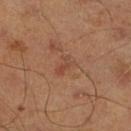workup = imaged on a skin check; not biopsied | subject = male, aged approximately 45 | acquisition = ~15 mm crop, total-body skin-cancer survey | TBP lesion metrics = a mean CIELAB color near L≈45 a*≈22 b*≈30, about 6 CIELAB-L* units darker than the surrounding skin, and a normalized lesion–skin contrast near 5; a within-lesion color-variation index near 0/10 and a peripheral color-asymmetry measure near 0 | lesion size = ≈2.5 mm | anatomic site = the right lower leg.